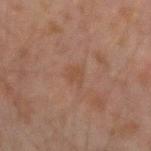The lesion was photographed on a routine skin check and not biopsied; there is no pathology result.
Imaged with cross-polarized lighting.
The lesion is on the left forearm.
A male subject, aged around 30.
The lesion-visualizer software estimated a lesion-to-skin contrast of about 4.5 (normalized; higher = more distinct). The analysis additionally found border irregularity of about 4 on a 0–10 scale and a within-lesion color-variation index near 1/10.
A 15 mm close-up extracted from a 3D total-body photography capture.